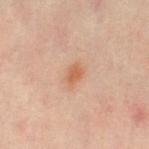Recorded during total-body skin imaging; not selected for excision or biopsy. This image is a 15 mm lesion crop taken from a total-body photograph. A female subject, aged approximately 40. Automated image analysis of the tile measured an area of roughly 3.5 mm², an outline eccentricity of about 0.75 (0 = round, 1 = elongated), and a symmetry-axis asymmetry near 0.2. The analysis additionally found about 9 CIELAB-L* units darker than the surrounding skin and a normalized border contrast of about 7. Longest diameter approximately 2.5 mm. Imaged with cross-polarized lighting. Located on the leg.